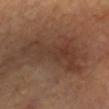follow-up = catalogued during a skin exam; not biopsied
size = ≈8 mm
image source = 15 mm crop, total-body photography
body site = the chest
patient = female, roughly 60 years of age
lighting = cross-polarized